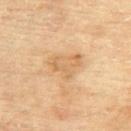Part of a total-body skin-imaging series; this lesion was reviewed on a skin check and was not flagged for biopsy. A female patient, about 80 years old. A region of skin cropped from a whole-body photographic capture, roughly 15 mm wide. An algorithmic analysis of the crop reported a border-irregularity index near 4.5/10, internal color variation of about 3 on a 0–10 scale, and radial color variation of about 1. It also reported an automated nevus-likeness rating near 0 out of 100 and a lesion-detection confidence of about 100/100. About 4 mm across. On the upper back.